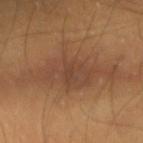follow-up — total-body-photography surveillance lesion; no biopsy | lighting — cross-polarized illumination | patient — male, approximately 60 years of age | lesion diameter — ~11 mm (longest diameter) | imaging modality — total-body-photography crop, ~15 mm field of view | anatomic site — the right upper arm.